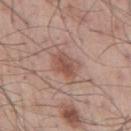<lesion>
<biopsy_status>not biopsied; imaged during a skin examination</biopsy_status>
<patient>
  <sex>male</sex>
  <age_approx>55</age_approx>
</patient>
<site>back</site>
<image>
  <source>total-body photography crop</source>
  <field_of_view_mm>15</field_of_view_mm>
</image>
</lesion>